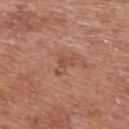Q: Is there a histopathology result?
A: catalogued during a skin exam; not biopsied
Q: What did automated image analysis measure?
A: a footprint of about 3.5 mm², an outline eccentricity of about 0.8 (0 = round, 1 = elongated), and a symmetry-axis asymmetry near 0.5; a normalized border contrast of about 5.5; a classifier nevus-likeness of about 0/100 and a detector confidence of about 100 out of 100 that the crop contains a lesion
Q: How was the tile lit?
A: white-light
Q: Who is the patient?
A: male, aged 68 to 72
Q: How was this image acquired?
A: ~15 mm crop, total-body skin-cancer survey
Q: Lesion location?
A: the upper back
Q: Lesion size?
A: about 2.5 mm